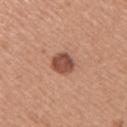A female subject in their mid- to late 30s. From the right upper arm. A close-up tile cropped from a whole-body skin photograph, about 15 mm across. Captured under white-light illumination.